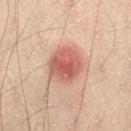workup = imaged on a skin check; not biopsied | imaging modality = ~15 mm crop, total-body skin-cancer survey | automated metrics = an area of roughly 15 mm²; radial color variation of about 1.5 | illumination = cross-polarized illumination | subject = male, aged 38–42 | size = about 5 mm | site = the right thigh.